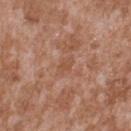This lesion was catalogued during total-body skin photography and was not selected for biopsy. The lesion's longest dimension is about 2.5 mm. Cropped from a total-body skin-imaging series; the visible field is about 15 mm. The subject is a male aged 43 to 47. Located on the upper back. This is a white-light tile.